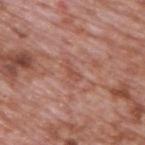Clinical impression: The lesion was tiled from a total-body skin photograph and was not biopsied. Context: About 2.5 mm across. This image is a 15 mm lesion crop taken from a total-body photograph. Imaged with white-light lighting. The lesion is on the upper back. A male patient, approximately 70 years of age. Automated tile analysis of the lesion measured peripheral color asymmetry of about 0. And it measured a classifier nevus-likeness of about 0/100 and a lesion-detection confidence of about 85/100.